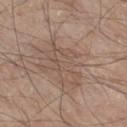The lesion was photographed on a routine skin check and not biopsied; there is no pathology result. A region of skin cropped from a whole-body photographic capture, roughly 15 mm wide. Automated tile analysis of the lesion measured a lesion color around L≈52 a*≈16 b*≈25 in CIELAB, a lesion–skin lightness drop of about 6, and a lesion-to-skin contrast of about 4.5 (normalized; higher = more distinct). The analysis additionally found a border-irregularity index near 8.5/10 and a peripheral color-asymmetry measure near 1. The software also gave a detector confidence of about 60 out of 100 that the crop contains a lesion. The lesion's longest dimension is about 6 mm. A male subject, about 80 years old. From the leg.